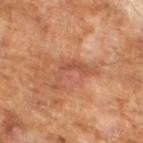Assessment:
No biopsy was performed on this lesion — it was imaged during a full skin examination and was not determined to be concerning.
Background:
The subject is a male aged 63–67. Cropped from a total-body skin-imaging series; the visible field is about 15 mm. The lesion-visualizer software estimated an area of roughly 6.5 mm², a shape eccentricity near 0.9, and two-axis asymmetry of about 0.75. The software also gave a border-irregularity index near 9.5/10 and radial color variation of about 0. The software also gave an automated nevus-likeness rating near 0 out of 100 and a detector confidence of about 90 out of 100 that the crop contains a lesion. About 5.5 mm across. The tile uses cross-polarized illumination.